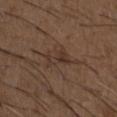A 15 mm crop from a total-body photograph taken for skin-cancer surveillance. About 3 mm across. The tile uses white-light illumination. A male subject roughly 50 years of age. The lesion-visualizer software estimated a footprint of about 4.5 mm². It also reported a nevus-likeness score of about 0/100 and a lesion-detection confidence of about 90/100. The lesion is on the chest.